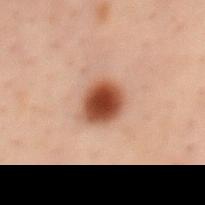<record>
  <biopsy_status>not biopsied; imaged during a skin examination</biopsy_status>
  <patient>
    <sex>male</sex>
    <age_approx>40</age_approx>
  </patient>
  <site>mid back</site>
  <image>
    <source>total-body photography crop</source>
    <field_of_view_mm>15</field_of_view_mm>
  </image>
</record>